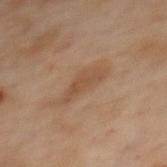Impression: The lesion was tiled from a total-body skin photograph and was not biopsied. Acquisition and patient details: Cropped from a whole-body photographic skin survey; the tile spans about 15 mm. The tile uses cross-polarized illumination. Longest diameter approximately 4 mm. Located on the back. An algorithmic analysis of the crop reported a border-irregularity rating of about 4.5/10 and a peripheral color-asymmetry measure near 0.5. A female subject, roughly 55 years of age.